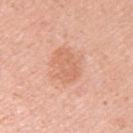notes = catalogued during a skin exam; not biopsied | acquisition = ~15 mm crop, total-body skin-cancer survey | diameter = about 4.5 mm | automated lesion analysis = border irregularity of about 3 on a 0–10 scale, a color-variation rating of about 2.5/10, and radial color variation of about 1 | location = the left upper arm | subject = female, aged around 30.